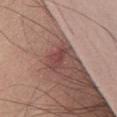The lesion was photographed on a routine skin check and not biopsied; there is no pathology result. A roughly 15 mm field-of-view crop from a total-body skin photograph. Measured at roughly 3.5 mm in maximum diameter. Imaged with white-light lighting. The lesion is located on the front of the torso. A male patient, aged 48–52.